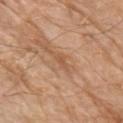Q: Was a biopsy performed?
A: no biopsy performed (imaged during a skin exam)
Q: What are the patient's age and sex?
A: male, approximately 80 years of age
Q: What is the lesion's diameter?
A: ≈2.5 mm
Q: How was the tile lit?
A: white-light
Q: Where on the body is the lesion?
A: the left upper arm
Q: What is the imaging modality?
A: ~15 mm tile from a whole-body skin photo